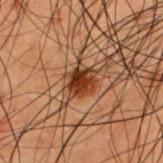{
  "biopsy_status": "not biopsied; imaged during a skin examination",
  "lesion_size": {
    "long_diameter_mm_approx": 3.5
  },
  "patient": {
    "sex": "male",
    "age_approx": 50
  },
  "site": "upper back",
  "image": {
    "source": "total-body photography crop",
    "field_of_view_mm": 15
  }
}